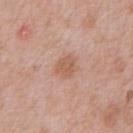Notes:
* workup — catalogued during a skin exam; not biopsied
* imaging modality — ~15 mm crop, total-body skin-cancer survey
* body site — the chest
* subject — male, aged 48 to 52
* size — about 3 mm
* TBP lesion metrics — a footprint of about 5 mm²; an average lesion color of about L≈58 a*≈21 b*≈30 (CIELAB), a lesion–skin lightness drop of about 8, and a normalized lesion–skin contrast near 6.5
* tile lighting — white-light